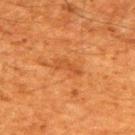Q: Who is the patient?
A: male, aged 58–62
Q: How was this image acquired?
A: total-body-photography crop, ~15 mm field of view
Q: Lesion location?
A: the back
Q: What is the lesion's diameter?
A: ≈3.5 mm
Q: Illumination type?
A: cross-polarized illumination